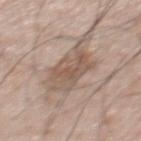Assessment: The lesion was photographed on a routine skin check and not biopsied; there is no pathology result. Context: A male patient, about 55 years old. A 15 mm close-up extracted from a 3D total-body photography capture. The lesion is on the back. The tile uses white-light illumination. The recorded lesion diameter is about 5 mm.